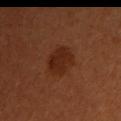Recorded during total-body skin imaging; not selected for excision or biopsy. Captured under cross-polarized illumination. Automated tile analysis of the lesion measured a lesion color around L≈26 a*≈23 b*≈29 in CIELAB, roughly 7 lightness units darker than nearby skin, and a lesion-to-skin contrast of about 7.5 (normalized; higher = more distinct). The lesion's longest dimension is about 3.5 mm. A region of skin cropped from a whole-body photographic capture, roughly 15 mm wide. A female subject aged 48 to 52.